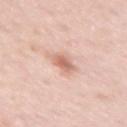- workup: imaged on a skin check; not biopsied
- automated metrics: an area of roughly 4.5 mm² and two-axis asymmetry of about 0.3; a border-irregularity index near 2.5/10; a classifier nevus-likeness of about 60/100 and a detector confidence of about 100 out of 100 that the crop contains a lesion
- location: the right upper arm
- imaging modality: 15 mm crop, total-body photography
- lighting: white-light
- diameter: ≈3 mm
- patient: female, roughly 40 years of age